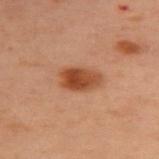{
  "biopsy_status": "not biopsied; imaged during a skin examination",
  "lighting": "cross-polarized",
  "automated_metrics": {
    "eccentricity": 0.8,
    "shape_asymmetry": 0.2,
    "nevus_likeness_0_100": 100,
    "lesion_detection_confidence_0_100": 100
  },
  "lesion_size": {
    "long_diameter_mm_approx": 4.5
  },
  "site": "upper back",
  "patient": {
    "sex": "female",
    "age_approx": 55
  },
  "image": {
    "source": "total-body photography crop",
    "field_of_view_mm": 15
  }
}